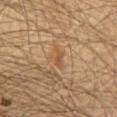Assessment:
Imaged during a routine full-body skin examination; the lesion was not biopsied and no histopathology is available.
Clinical summary:
Cropped from a whole-body photographic skin survey; the tile spans about 15 mm. This is a cross-polarized tile. A male subject, about 70 years old. An algorithmic analysis of the crop reported an outline eccentricity of about 0.9 (0 = round, 1 = elongated) and a shape-asymmetry score of about 0.5 (0 = symmetric). The software also gave an average lesion color of about L≈52 a*≈18 b*≈35 (CIELAB), about 7 CIELAB-L* units darker than the surrounding skin, and a normalized border contrast of about 5.5. And it measured a nevus-likeness score of about 0/100. From the chest. About 2.5 mm across.